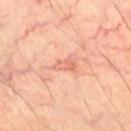biopsy status: total-body-photography surveillance lesion; no biopsy
TBP lesion metrics: a border-irregularity rating of about 4/10
size: ≈2.5 mm
patient: male, aged 58–62
imaging modality: 15 mm crop, total-body photography
site: the leg
illumination: cross-polarized illumination